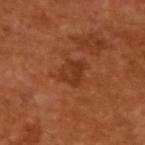Impression: Captured during whole-body skin photography for melanoma surveillance; the lesion was not biopsied. Context: The lesion's longest dimension is about 3 mm. The patient is a male approximately 65 years of age. A close-up tile cropped from a whole-body skin photograph, about 15 mm across.